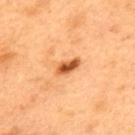follow-up — imaged on a skin check; not biopsied | TBP lesion metrics — a lesion area of about 4 mm² and an outline eccentricity of about 0.85 (0 = round, 1 = elongated); a border-irregularity rating of about 3/10, a within-lesion color-variation index near 3/10, and peripheral color asymmetry of about 1 | subject — male, aged approximately 50 | site — the upper back | size — ~3 mm (longest diameter) | acquisition — ~15 mm tile from a whole-body skin photo | lighting — cross-polarized.